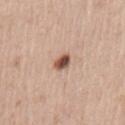Assessment:
The lesion was tiled from a total-body skin photograph and was not biopsied.
Image and clinical context:
Automated image analysis of the tile measured a shape eccentricity near 0.7. It also reported a lesion color around L≈52 a*≈21 b*≈28 in CIELAB and a normalized border contrast of about 11.5. Located on the chest. A roughly 15 mm field-of-view crop from a total-body skin photograph. Captured under white-light illumination. A male subject about 65 years old. The recorded lesion diameter is about 2.5 mm.